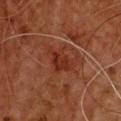Part of a total-body skin-imaging series; this lesion was reviewed on a skin check and was not flagged for biopsy. Approximately 4 mm at its widest. The subject is a male aged 58 to 62. A lesion tile, about 15 mm wide, cut from a 3D total-body photograph. Automated image analysis of the tile measured a lesion area of about 7.5 mm² and a shape-asymmetry score of about 0.35 (0 = symmetric). The software also gave a mean CIELAB color near L≈26 a*≈24 b*≈27 and about 6 CIELAB-L* units darker than the surrounding skin. The analysis additionally found a border-irregularity index near 4/10 and a within-lesion color-variation index near 3/10. It also reported a lesion-detection confidence of about 100/100. On the chest.